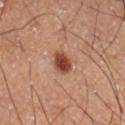biopsy status — total-body-photography surveillance lesion; no biopsy | size — ≈3 mm | acquisition — ~15 mm crop, total-body skin-cancer survey | lighting — cross-polarized illumination | location — the left thigh | patient — male, aged 58 to 62 | automated metrics — an area of roughly 5.5 mm² and a shape-asymmetry score of about 0.2 (0 = symmetric); a mean CIELAB color near L≈41 a*≈22 b*≈28, about 14 CIELAB-L* units darker than the surrounding skin, and a lesion-to-skin contrast of about 11 (normalized; higher = more distinct); radial color variation of about 1; a classifier nevus-likeness of about 100/100 and lesion-presence confidence of about 100/100.